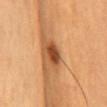| key | value |
|---|---|
| notes | total-body-photography surveillance lesion; no biopsy |
| diameter | about 3 mm |
| patient | male, about 60 years old |
| TBP lesion metrics | an area of roughly 4.5 mm², an eccentricity of roughly 0.8, and a symmetry-axis asymmetry near 0.2; a border-irregularity rating of about 2/10, a color-variation rating of about 4/10, and peripheral color asymmetry of about 1.5; a nevus-likeness score of about 95/100 and a lesion-detection confidence of about 100/100 |
| location | the back |
| lighting | cross-polarized illumination |
| image | ~15 mm tile from a whole-body skin photo |